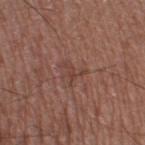biopsy status: no biopsy performed (imaged during a skin exam) | subject: male, approximately 50 years of age | location: the right thigh | lighting: white-light illumination | acquisition: 15 mm crop, total-body photography | TBP lesion metrics: a footprint of about 3 mm² and an outline eccentricity of about 0.6 (0 = round, 1 = elongated); a lesion-to-skin contrast of about 5 (normalized; higher = more distinct); a border-irregularity rating of about 9/10, internal color variation of about 0 on a 0–10 scale, and peripheral color asymmetry of about 0.